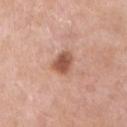<lesion>
  <biopsy_status>not biopsied; imaged during a skin examination</biopsy_status>
  <site>chest</site>
  <image>
    <source>total-body photography crop</source>
    <field_of_view_mm>15</field_of_view_mm>
  </image>
  <lighting>white-light</lighting>
  <patient>
    <sex>male</sex>
    <age_approx>55</age_approx>
  </patient>
  <automated_metrics>
    <area_mm2_approx>5.5</area_mm2_approx>
    <eccentricity>0.35</eccentricity>
    <cielab_L>54</cielab_L>
    <cielab_a>23</cielab_a>
    <cielab_b>31</cielab_b>
    <vs_skin_darker_L>14.0</vs_skin_darker_L>
    <vs_skin_contrast_norm>9.0</vs_skin_contrast_norm>
    <border_irregularity_0_10>2.0</border_irregularity_0_10>
    <color_variation_0_10>2.5</color_variation_0_10>
    <peripheral_color_asymmetry>1.0</peripheral_color_asymmetry>
    <nevus_likeness_0_100>90</nevus_likeness_0_100>
    <lesion_detection_confidence_0_100>100</lesion_detection_confidence_0_100>
  </automated_metrics>
</lesion>